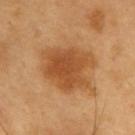follow-up: total-body-photography surveillance lesion; no biopsy
tile lighting: cross-polarized illumination
subject: male, aged approximately 60
anatomic site: the upper back
size: ~6 mm (longest diameter)
image: ~15 mm crop, total-body skin-cancer survey
image-analysis metrics: an outline eccentricity of about 0.5 (0 = round, 1 = elongated) and two-axis asymmetry of about 0.25; an average lesion color of about L≈50 a*≈24 b*≈40 (CIELAB), roughly 11 lightness units darker than nearby skin, and a normalized border contrast of about 8; a border-irregularity rating of about 3/10, a within-lesion color-variation index near 3.5/10, and radial color variation of about 1